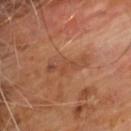Q: Was this lesion biopsied?
A: imaged on a skin check; not biopsied
Q: What lighting was used for the tile?
A: cross-polarized
Q: Patient demographics?
A: male, aged around 60
Q: What is the imaging modality?
A: 15 mm crop, total-body photography
Q: What is the anatomic site?
A: the upper back
Q: What is the lesion's diameter?
A: ~5 mm (longest diameter)